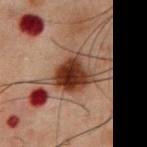imaging modality=~15 mm tile from a whole-body skin photo | site=the chest | diameter=~5 mm (longest diameter) | subject=male, aged around 60 | illumination=cross-polarized illumination | automated metrics=an average lesion color of about L≈29 a*≈18 b*≈24 (CIELAB), a lesion–skin lightness drop of about 13, and a normalized border contrast of about 12.5; a border-irregularity index near 3/10, a within-lesion color-variation index near 8/10, and a peripheral color-asymmetry measure near 2.5.